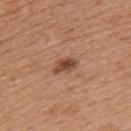Findings:
* subject · female, about 40 years old
* size · ≈3 mm
* image source · 15 mm crop, total-body photography
* lighting · white-light
* automated lesion analysis · a footprint of about 4.5 mm²; roughly 12 lightness units darker than nearby skin and a lesion-to-skin contrast of about 9 (normalized; higher = more distinct); a classifier nevus-likeness of about 75/100 and a lesion-detection confidence of about 100/100
* body site · the left upper arm